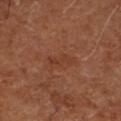Findings:
• follow-up: catalogued during a skin exam; not biopsied
• location: the leg
• size: ~3 mm (longest diameter)
• automated metrics: an average lesion color of about L≈37 a*≈24 b*≈31 (CIELAB) and a normalized lesion–skin contrast near 5
• subject: aged approximately 65
• illumination: cross-polarized
• acquisition: 15 mm crop, total-body photography